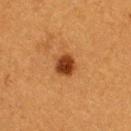Notes:
– notes — imaged on a skin check; not biopsied
– imaging modality — 15 mm crop, total-body photography
– lighting — cross-polarized illumination
– anatomic site — the upper back
– diameter — about 2.5 mm
– patient — female, in their mid-50s